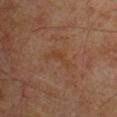Q: Was this lesion biopsied?
A: catalogued during a skin exam; not biopsied
Q: What is the imaging modality?
A: ~15 mm crop, total-body skin-cancer survey
Q: Lesion location?
A: the upper back
Q: Patient demographics?
A: male, aged around 60
Q: How was the tile lit?
A: cross-polarized illumination